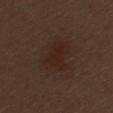Captured during whole-body skin photography for melanoma surveillance; the lesion was not biopsied.
The patient is a male roughly 70 years of age.
Captured under white-light illumination.
Measured at roughly 4.5 mm in maximum diameter.
A 15 mm close-up extracted from a 3D total-body photography capture.
Automated image analysis of the tile measured an area of roughly 15 mm² and a symmetry-axis asymmetry near 0.25. And it measured a border-irregularity rating of about 3.5/10, internal color variation of about 2.5 on a 0–10 scale, and radial color variation of about 1. And it measured a nevus-likeness score of about 45/100 and lesion-presence confidence of about 100/100.
On the leg.